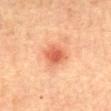Recorded during total-body skin imaging; not selected for excision or biopsy.
A female subject about 60 years old.
A lesion tile, about 15 mm wide, cut from a 3D total-body photograph.
On the abdomen.